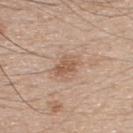Recorded during total-body skin imaging; not selected for excision or biopsy. A male subject approximately 50 years of age. An algorithmic analysis of the crop reported a footprint of about 6.5 mm² and a shape-asymmetry score of about 0.3 (0 = symmetric). The software also gave border irregularity of about 4 on a 0–10 scale, a color-variation rating of about 3/10, and radial color variation of about 1. A 15 mm crop from a total-body photograph taken for skin-cancer surveillance. The lesion's longest dimension is about 3.5 mm. The lesion is located on the upper back. Imaged with white-light lighting.